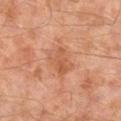  biopsy_status: not biopsied; imaged during a skin examination
  site: right lower leg
  patient:
    sex: male
    age_approx: 60
  lesion_size:
    long_diameter_mm_approx: 4.0
  image:
    source: total-body photography crop
    field_of_view_mm: 15
  lighting: cross-polarized
  automated_metrics:
    area_mm2_approx: 9.0
    eccentricity: 0.7
    shape_asymmetry: 0.25
    cielab_L: 53
    cielab_a: 24
    cielab_b: 33
    vs_skin_darker_L: 7.0
    vs_skin_contrast_norm: 5.5
    border_irregularity_0_10: 2.5
    color_variation_0_10: 4.0
    peripheral_color_asymmetry: 1.5